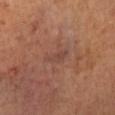The lesion was photographed on a routine skin check and not biopsied; there is no pathology result. A region of skin cropped from a whole-body photographic capture, roughly 15 mm wide. Imaged with cross-polarized lighting. Located on the leg. Measured at roughly 3.5 mm in maximum diameter. The subject is a female in their mid- to late 60s.